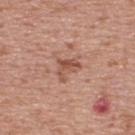This lesion was catalogued during total-body skin photography and was not selected for biopsy. From the upper back. A roughly 15 mm field-of-view crop from a total-body skin photograph. This is a white-light tile. A male patient aged 68–72. Automated tile analysis of the lesion measured a lesion–skin lightness drop of about 10 and a normalized lesion–skin contrast near 7. Longest diameter approximately 3 mm.